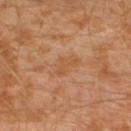<tbp_lesion>
<biopsy_status>not biopsied; imaged during a skin examination</biopsy_status>
<image>
  <source>total-body photography crop</source>
  <field_of_view_mm>15</field_of_view_mm>
</image>
<patient>
  <sex>male</sex>
  <age_approx>30</age_approx>
</patient>
<site>leg</site>
<lighting>cross-polarized</lighting>
<lesion_size>
  <long_diameter_mm_approx>3.0</long_diameter_mm_approx>
</lesion_size>
</tbp_lesion>